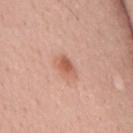| key | value |
|---|---|
| biopsy status | total-body-photography surveillance lesion; no biopsy |
| patient | male, aged 53–57 |
| image | 15 mm crop, total-body photography |